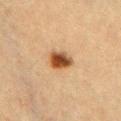biopsy_status: not biopsied; imaged during a skin examination
site: chest
lighting: cross-polarized
image:
  source: total-body photography crop
  field_of_view_mm: 15
automated_metrics:
  border_irregularity_0_10: 2.0
  color_variation_0_10: 6.0
  peripheral_color_asymmetry: 1.5
lesion_size:
  long_diameter_mm_approx: 3.5
patient:
  sex: female
  age_approx: 55